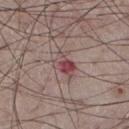<tbp_lesion>
  <biopsy_status>not biopsied; imaged during a skin examination</biopsy_status>
  <lighting>white-light</lighting>
  <lesion_size>
    <long_diameter_mm_approx>3.5</long_diameter_mm_approx>
  </lesion_size>
  <automated_metrics>
    <cielab_L>47</cielab_L>
    <cielab_a>22</cielab_a>
    <cielab_b>18</cielab_b>
    <vs_skin_darker_L>10.0</vs_skin_darker_L>
    <vs_skin_contrast_norm>8.0</vs_skin_contrast_norm>
    <border_irregularity_0_10>3.5</border_irregularity_0_10>
    <color_variation_0_10>8.5</color_variation_0_10>
    <peripheral_color_asymmetry>2.5</peripheral_color_asymmetry>
    <lesion_detection_confidence_0_100>100</lesion_detection_confidence_0_100>
  </automated_metrics>
  <image>
    <source>total-body photography crop</source>
    <field_of_view_mm>15</field_of_view_mm>
  </image>
  <patient>
    <sex>male</sex>
    <age_approx>75</age_approx>
  </patient>
  <site>left thigh</site>
</tbp_lesion>